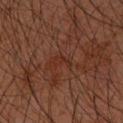Part of a total-body skin-imaging series; this lesion was reviewed on a skin check and was not flagged for biopsy.
A 15 mm close-up tile from a total-body photography series done for melanoma screening.
The subject is a male aged around 60.
From the left forearm.
The recorded lesion diameter is about 2.5 mm.
The tile uses cross-polarized illumination.